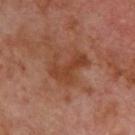The lesion was photographed on a routine skin check and not biopsied; there is no pathology result. The lesion is located on the back. Cropped from a total-body skin-imaging series; the visible field is about 15 mm. Automated tile analysis of the lesion measured a border-irregularity rating of about 6.5/10. It also reported a lesion-detection confidence of about 100/100. Measured at roughly 5 mm in maximum diameter. The patient is a male aged approximately 70.